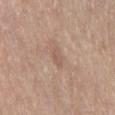The lesion was tiled from a total-body skin photograph and was not biopsied.
A male subject roughly 65 years of age.
The tile uses white-light illumination.
The lesion-visualizer software estimated a mean CIELAB color near L≈56 a*≈18 b*≈27, about 7 CIELAB-L* units darker than the surrounding skin, and a normalized border contrast of about 5. The software also gave an automated nevus-likeness rating near 0 out of 100 and a lesion-detection confidence of about 100/100.
A 15 mm crop from a total-body photograph taken for skin-cancer surveillance.
The lesion is on the abdomen.